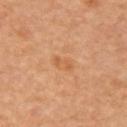Assessment: This lesion was catalogued during total-body skin photography and was not selected for biopsy. Context: A male subject, aged approximately 85 or older. This image is a 15 mm lesion crop taken from a total-body photograph. From the right upper arm.